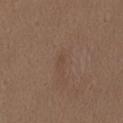{
  "image": {
    "source": "total-body photography crop",
    "field_of_view_mm": 15
  },
  "patient": {
    "sex": "female",
    "age_approx": 40
  },
  "lighting": "white-light",
  "site": "abdomen",
  "lesion_size": {
    "long_diameter_mm_approx": 1.5
  },
  "automated_metrics": {
    "area_mm2_approx": 1.0,
    "eccentricity": 0.85,
    "shape_asymmetry": 0.25,
    "cielab_L": 44,
    "cielab_a": 17,
    "cielab_b": 27,
    "vs_skin_darker_L": 4.0,
    "vs_skin_contrast_norm": 4.5,
    "border_irregularity_0_10": 2.0,
    "color_variation_0_10": 0.0,
    "peripheral_color_asymmetry": 0.0
  }
}